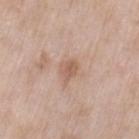• workup — total-body-photography surveillance lesion; no biopsy
• illumination — white-light
• anatomic site — the leg
• lesion diameter — ≈2.5 mm
• patient — female, aged 73 to 77
• acquisition — total-body-photography crop, ~15 mm field of view